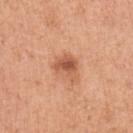{
  "biopsy_status": "not biopsied; imaged during a skin examination",
  "lighting": "white-light",
  "site": "arm",
  "lesion_size": {
    "long_diameter_mm_approx": 3.0
  },
  "automated_metrics": {
    "cielab_L": 57,
    "cielab_a": 27,
    "cielab_b": 35,
    "vs_skin_darker_L": 11.0,
    "vs_skin_contrast_norm": 7.5,
    "border_irregularity_0_10": 4.0,
    "color_variation_0_10": 4.0,
    "nevus_likeness_0_100": 80,
    "lesion_detection_confidence_0_100": 100
  },
  "image": {
    "source": "total-body photography crop",
    "field_of_view_mm": 15
  },
  "patient": {
    "sex": "female",
    "age_approx": 50
  }
}